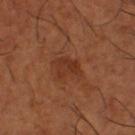Clinical impression:
Recorded during total-body skin imaging; not selected for excision or biopsy.
Background:
A close-up tile cropped from a whole-body skin photograph, about 15 mm across. About 2.5 mm across. An algorithmic analysis of the crop reported a lesion area of about 5.5 mm², a shape eccentricity near 0.6, and two-axis asymmetry of about 0.4. It also reported a mean CIELAB color near L≈34 a*≈24 b*≈31, roughly 7 lightness units darker than nearby skin, and a lesion-to-skin contrast of about 6 (normalized; higher = more distinct). It also reported a border-irregularity rating of about 4/10 and internal color variation of about 3 on a 0–10 scale. The tile uses cross-polarized illumination. The subject is a male aged approximately 65. From the leg.